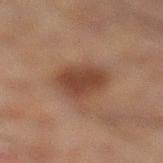Clinical impression: This lesion was catalogued during total-body skin photography and was not selected for biopsy. Background: This is a cross-polarized tile. Approximately 4 mm at its widest. The subject is a male approximately 60 years of age. Located on the leg. The lesion-visualizer software estimated an area of roughly 12 mm², an eccentricity of roughly 0.4, and a symmetry-axis asymmetry near 0.2. It also reported a border-irregularity index near 2/10, internal color variation of about 2 on a 0–10 scale, and radial color variation of about 0.5. And it measured an automated nevus-likeness rating near 95 out of 100 and a lesion-detection confidence of about 100/100. A 15 mm crop from a total-body photograph taken for skin-cancer surveillance.